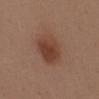A male subject aged 38–42.
The lesion is located on the mid back.
The tile uses white-light illumination.
The recorded lesion diameter is about 4.5 mm.
A roughly 15 mm field-of-view crop from a total-body skin photograph.